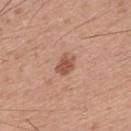Impression:
The lesion was photographed on a routine skin check and not biopsied; there is no pathology result.
Image and clinical context:
A male subject aged 28 to 32. The lesion is located on the upper back. This is a white-light tile. The total-body-photography lesion software estimated an area of roughly 4 mm², an eccentricity of roughly 0.6, and a shape-asymmetry score of about 0.2 (0 = symmetric). The analysis additionally found a normalized lesion–skin contrast near 8. The software also gave internal color variation of about 1.5 on a 0–10 scale and peripheral color asymmetry of about 0.5. The analysis additionally found an automated nevus-likeness rating near 60 out of 100. A region of skin cropped from a whole-body photographic capture, roughly 15 mm wide.